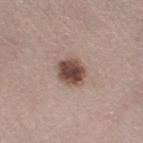biopsy_status: not biopsied; imaged during a skin examination
image:
  source: total-body photography crop
  field_of_view_mm: 15
site: left thigh
lesion_size:
  long_diameter_mm_approx: 3.5
patient:
  sex: female
  age_approx: 50